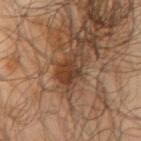  biopsy_status: not biopsied; imaged during a skin examination
  patient:
    sex: male
    age_approx: 65
  lighting: cross-polarized
  lesion_size:
    long_diameter_mm_approx: 4.0
  image:
    source: total-body photography crop
    field_of_view_mm: 15
  site: right upper arm